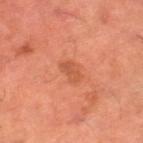– TBP lesion metrics: an average lesion color of about L≈52 a*≈29 b*≈36 (CIELAB) and about 7 CIELAB-L* units darker than the surrounding skin; border irregularity of about 2.5 on a 0–10 scale, a color-variation rating of about 2/10, and radial color variation of about 0.5; a nevus-likeness score of about 25/100 and lesion-presence confidence of about 100/100
– image source: ~15 mm tile from a whole-body skin photo
– subject: male, aged around 60
– body site: the leg